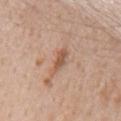Recorded during total-body skin imaging; not selected for excision or biopsy. The lesion's longest dimension is about 4 mm. On the mid back. The subject is a male approximately 65 years of age. This is a white-light tile. A close-up tile cropped from a whole-body skin photograph, about 15 mm across.